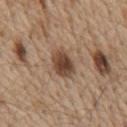The tile uses white-light illumination.
The lesion is located on the chest.
A region of skin cropped from a whole-body photographic capture, roughly 15 mm wide.
Approximately 4 mm at its widest.
The subject is a male aged around 70.